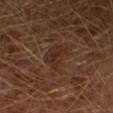notes: catalogued during a skin exam; not biopsied | body site: the left leg | subject: male, roughly 30 years of age | tile lighting: cross-polarized | size: ≈4 mm | automated metrics: a border-irregularity index near 4/10, a color-variation rating of about 3/10, and radial color variation of about 1; an automated nevus-likeness rating near 0 out of 100 and a lesion-detection confidence of about 65/100 | acquisition: total-body-photography crop, ~15 mm field of view.